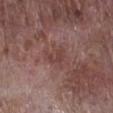No biopsy was performed on this lesion — it was imaged during a full skin examination and was not determined to be concerning. Longest diameter approximately 2.5 mm. Cropped from a whole-body photographic skin survey; the tile spans about 15 mm. This is a white-light tile. A male patient, roughly 80 years of age. On the right lower leg.